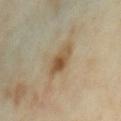Part of a total-body skin-imaging series; this lesion was reviewed on a skin check and was not flagged for biopsy. A 15 mm close-up extracted from a 3D total-body photography capture. Located on the chest. The patient is a female roughly 35 years of age. The total-body-photography lesion software estimated a footprint of about 8 mm². It also reported a mean CIELAB color near L≈51 a*≈13 b*≈33. The tile uses cross-polarized illumination. The lesion's longest dimension is about 4.5 mm.